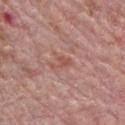Clinical impression: The lesion was photographed on a routine skin check and not biopsied; there is no pathology result. Context: This is a white-light tile. A close-up tile cropped from a whole-body skin photograph, about 15 mm across. The lesion is on the chest. A male patient, approximately 70 years of age. About 3 mm across. The lesion-visualizer software estimated an area of roughly 3.5 mm² and two-axis asymmetry of about 0.45. And it measured a lesion color around L≈53 a*≈23 b*≈27 in CIELAB, roughly 7 lightness units darker than nearby skin, and a lesion-to-skin contrast of about 5.5 (normalized; higher = more distinct). It also reported border irregularity of about 5 on a 0–10 scale, a color-variation rating of about 0.5/10, and peripheral color asymmetry of about 0.5. And it measured an automated nevus-likeness rating near 0 out of 100 and lesion-presence confidence of about 100/100.